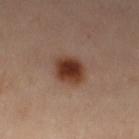biopsy_status: not biopsied; imaged during a skin examination
patient:
  sex: female
  age_approx: 40
lighting: cross-polarized
lesion_size:
  long_diameter_mm_approx: 3.5
image:
  source: total-body photography crop
  field_of_view_mm: 15
site: right leg